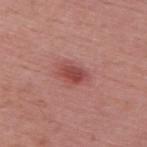The lesion was tiled from a total-body skin photograph and was not biopsied. A male patient, aged 53 to 57. The lesion is on the upper back. Cropped from a total-body skin-imaging series; the visible field is about 15 mm.